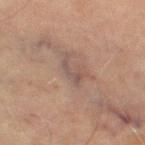* workup — total-body-photography surveillance lesion; no biopsy
* subject — male, roughly 70 years of age
* illumination — cross-polarized
* diameter — ≈3 mm
* automated metrics — an area of roughly 2.5 mm², a shape eccentricity near 0.9, and a shape-asymmetry score of about 0.7 (0 = symmetric); an average lesion color of about L≈41 a*≈14 b*≈18 (CIELAB), a lesion–skin lightness drop of about 6, and a lesion-to-skin contrast of about 6 (normalized; higher = more distinct); border irregularity of about 9 on a 0–10 scale, a color-variation rating of about 0/10, and peripheral color asymmetry of about 0; a classifier nevus-likeness of about 0/100
* image source — ~15 mm crop, total-body skin-cancer survey
* body site — the right thigh